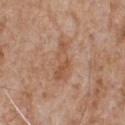Clinical impression:
The lesion was tiled from a total-body skin photograph and was not biopsied.
Background:
A male patient in their mid- to late 60s. Located on the front of the torso. A 15 mm close-up extracted from a 3D total-body photography capture.